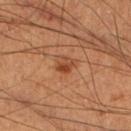The lesion was tiled from a total-body skin photograph and was not biopsied.
The subject is a male in their 60s.
A 15 mm close-up tile from a total-body photography series done for melanoma screening.
Measured at roughly 2.5 mm in maximum diameter.
On the right lower leg.